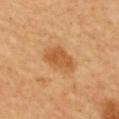Clinical impression: Part of a total-body skin-imaging series; this lesion was reviewed on a skin check and was not flagged for biopsy. Clinical summary: Captured under cross-polarized illumination. Approximately 4.5 mm at its widest. Cropped from a whole-body photographic skin survey; the tile spans about 15 mm. From the upper back. A female subject roughly 60 years of age.